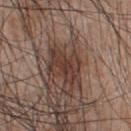biopsy status = catalogued during a skin exam; not biopsied
illumination = white-light illumination
body site = the upper back
subject = male, aged 43 to 47
acquisition = ~15 mm tile from a whole-body skin photo